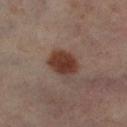Captured during whole-body skin photography for melanoma surveillance; the lesion was not biopsied.
A female subject, in their 50s.
Cropped from a whole-body photographic skin survey; the tile spans about 15 mm.
Located on the left lower leg.